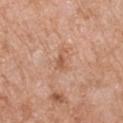No biopsy was performed on this lesion — it was imaged during a full skin examination and was not determined to be concerning.
The lesion is located on the left upper arm.
The lesion-visualizer software estimated an eccentricity of roughly 0.85. The analysis additionally found a border-irregularity index near 5/10, a color-variation rating of about 1/10, and peripheral color asymmetry of about 0. The analysis additionally found a classifier nevus-likeness of about 0/100 and a detector confidence of about 100 out of 100 that the crop contains a lesion.
A male subject, in their 60s.
Imaged with white-light lighting.
A 15 mm close-up tile from a total-body photography series done for melanoma screening.